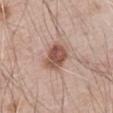Impression: The lesion was tiled from a total-body skin photograph and was not biopsied. Image and clinical context: This image is a 15 mm lesion crop taken from a total-body photograph. Automated tile analysis of the lesion measured a footprint of about 8 mm², an outline eccentricity of about 0.55 (0 = round, 1 = elongated), and a shape-asymmetry score of about 0.15 (0 = symmetric). The analysis additionally found a border-irregularity index near 2/10, a color-variation rating of about 4.5/10, and radial color variation of about 1.5. Measured at roughly 3.5 mm in maximum diameter. Captured under white-light illumination. The subject is a male aged approximately 70. From the right upper arm.